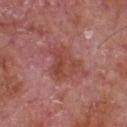Case summary:
* acquisition · ~15 mm crop, total-body skin-cancer survey
* subject · male, aged approximately 65
* body site · the chest